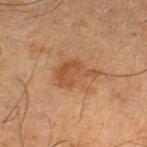notes: catalogued during a skin exam; not biopsied | subject: male, aged 63–67 | lighting: cross-polarized illumination | body site: the right thigh | lesion diameter: about 5 mm | image-analysis metrics: a lesion color around L≈41 a*≈19 b*≈30 in CIELAB and about 7 CIELAB-L* units darker than the surrounding skin | image source: ~15 mm tile from a whole-body skin photo.